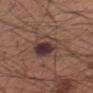{"biopsy_status": "not biopsied; imaged during a skin examination", "lesion_size": {"long_diameter_mm_approx": 4.5}, "image": {"source": "total-body photography crop", "field_of_view_mm": 15}, "lighting": "white-light", "site": "right forearm", "patient": {"sex": "male", "age_approx": 55}, "automated_metrics": {"cielab_L": 37, "cielab_a": 17, "cielab_b": 20, "border_irregularity_0_10": 1.5, "color_variation_0_10": 10.0, "peripheral_color_asymmetry": 3.0, "lesion_detection_confidence_0_100": 100}}